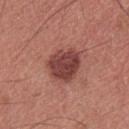Clinical summary:
Captured under white-light illumination. On the chest. A 15 mm close-up extracted from a 3D total-body photography capture. About 4.5 mm across. A male patient aged 33–37.